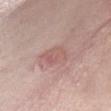Clinical impression:
Imaged during a routine full-body skin examination; the lesion was not biopsied and no histopathology is available.
Clinical summary:
This is a white-light tile. A female subject aged approximately 40. About 3.5 mm across. From the front of the torso. A 15 mm close-up extracted from a 3D total-body photography capture.